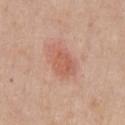Recorded during total-body skin imaging; not selected for excision or biopsy. A lesion tile, about 15 mm wide, cut from a 3D total-body photograph. From the chest. Approximately 4.5 mm at its widest. Automated tile analysis of the lesion measured a footprint of about 9.5 mm², a shape eccentricity near 0.8, and two-axis asymmetry of about 0.25. The software also gave roughly 8 lightness units darker than nearby skin and a normalized lesion–skin contrast near 6. The patient is a male aged approximately 65. The tile uses white-light illumination.